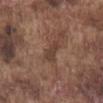{"biopsy_status": "not biopsied; imaged during a skin examination", "patient": {"sex": "male", "age_approx": 75}, "site": "front of the torso", "image": {"source": "total-body photography crop", "field_of_view_mm": 15}}